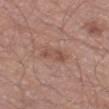Recorded during total-body skin imaging; not selected for excision or biopsy. The tile uses white-light illumination. The patient is a male about 70 years old. On the left thigh. Cropped from a whole-body photographic skin survey; the tile spans about 15 mm. Longest diameter approximately 3.5 mm.